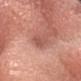Assessment: Captured during whole-body skin photography for melanoma surveillance; the lesion was not biopsied. Context: The patient is a male approximately 40 years of age. The recorded lesion diameter is about 3 mm. The lesion is located on the head or neck. A 15 mm crop from a total-body photograph taken for skin-cancer surveillance. The tile uses white-light illumination.